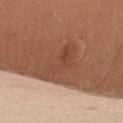<record>
<biopsy_status>not biopsied; imaged during a skin examination</biopsy_status>
<site>right upper arm</site>
<patient>
  <sex>female</sex>
  <age_approx>55</age_approx>
</patient>
<lesion_size>
  <long_diameter_mm_approx>4.5</long_diameter_mm_approx>
</lesion_size>
<image>
  <source>total-body photography crop</source>
  <field_of_view_mm>15</field_of_view_mm>
</image>
<lighting>white-light</lighting>
</record>